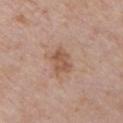Assessment: Part of a total-body skin-imaging series; this lesion was reviewed on a skin check and was not flagged for biopsy. Clinical summary: Automated image analysis of the tile measured an area of roughly 5.5 mm² and a symmetry-axis asymmetry near 0.3. And it measured a border-irregularity rating of about 3/10, internal color variation of about 2.5 on a 0–10 scale, and peripheral color asymmetry of about 1. The analysis additionally found an automated nevus-likeness rating near 15 out of 100. The recorded lesion diameter is about 3.5 mm. A female subject, in their 70s. A region of skin cropped from a whole-body photographic capture, roughly 15 mm wide. On the chest. The tile uses white-light illumination.